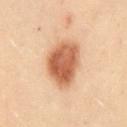Q: Was a biopsy performed?
A: no biopsy performed (imaged during a skin exam)
Q: What is the imaging modality?
A: 15 mm crop, total-body photography
Q: How was the tile lit?
A: cross-polarized illumination
Q: Where on the body is the lesion?
A: the mid back
Q: What did automated image analysis measure?
A: a shape eccentricity near 0.75; a mean CIELAB color near L≈48 a*≈20 b*≈30, roughly 14 lightness units darker than nearby skin, and a normalized lesion–skin contrast near 10; a border-irregularity rating of about 2/10 and a within-lesion color-variation index near 4.5/10
Q: Patient demographics?
A: female, aged approximately 30